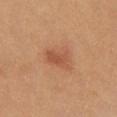notes — total-body-photography surveillance lesion; no biopsy